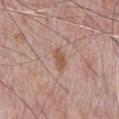<tbp_lesion>
<biopsy_status>not biopsied; imaged during a skin examination</biopsy_status>
<patient>
  <sex>male</sex>
  <age_approx>70</age_approx>
</patient>
<image>
  <source>total-body photography crop</source>
  <field_of_view_mm>15</field_of_view_mm>
</image>
<site>chest</site>
</tbp_lesion>